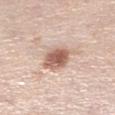Captured during whole-body skin photography for melanoma surveillance; the lesion was not biopsied.
A 15 mm close-up extracted from a 3D total-body photography capture.
A female patient, about 65 years old.
On the right lower leg.